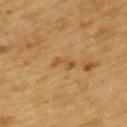Assessment:
No biopsy was performed on this lesion — it was imaged during a full skin examination and was not determined to be concerning.
Acquisition and patient details:
About 2.5 mm across. Cropped from a whole-body photographic skin survey; the tile spans about 15 mm. The total-body-photography lesion software estimated a mean CIELAB color near L≈45 a*≈17 b*≈37 and a normalized border contrast of about 5.5. The software also gave a border-irregularity index near 5.5/10 and a peripheral color-asymmetry measure near 0. Imaged with cross-polarized lighting. The patient is a male roughly 85 years of age or older. The lesion is on the upper back.